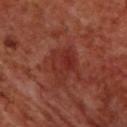Findings:
– workup · no biopsy performed (imaged during a skin exam)
– size · about 4.5 mm
– tile lighting · cross-polarized illumination
– image source · ~15 mm tile from a whole-body skin photo
– site · the back
– patient · male, aged 68 to 72
– automated metrics · an outline eccentricity of about 0.65 (0 = round, 1 = elongated) and two-axis asymmetry of about 0.35; an average lesion color of about L≈30 a*≈28 b*≈27 (CIELAB), about 6 CIELAB-L* units darker than the surrounding skin, and a normalized border contrast of about 6; a classifier nevus-likeness of about 0/100 and lesion-presence confidence of about 100/100